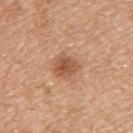* follow-up — imaged on a skin check; not biopsied
* patient — female, in their mid- to late 50s
* body site — the upper back
* lighting — white-light illumination
* image source — 15 mm crop, total-body photography
* diameter — ~3 mm (longest diameter)
* TBP lesion metrics — an automated nevus-likeness rating near 85 out of 100 and a lesion-detection confidence of about 100/100